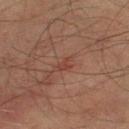Q: Was a biopsy performed?
A: total-body-photography surveillance lesion; no biopsy
Q: What is the anatomic site?
A: the left thigh
Q: Who is the patient?
A: male, aged approximately 75
Q: How was this image acquired?
A: 15 mm crop, total-body photography